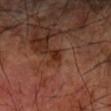Findings:
• biopsy status: no biopsy performed (imaged during a skin exam)
• patient: male, aged approximately 70
• image: ~15 mm tile from a whole-body skin photo
• site: the right forearm